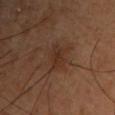Impression: Part of a total-body skin-imaging series; this lesion was reviewed on a skin check and was not flagged for biopsy. Clinical summary: On the chest. Cropped from a total-body skin-imaging series; the visible field is about 15 mm. A male subject, aged 58 to 62. The recorded lesion diameter is about 3 mm.